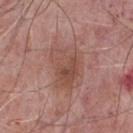workup: total-body-photography surveillance lesion; no biopsy | subject: male, approximately 75 years of age | lesion diameter: ≈6.5 mm | tile lighting: white-light illumination | image source: total-body-photography crop, ~15 mm field of view | location: the chest | automated metrics: a lesion–skin lightness drop of about 7.